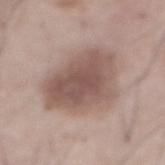{
  "lighting": "white-light",
  "image": {
    "source": "total-body photography crop",
    "field_of_view_mm": 15
  },
  "patient": {
    "sex": "male",
    "age_approx": 55
  },
  "site": "abdomen",
  "lesion_size": {
    "long_diameter_mm_approx": 8.0
  }
}